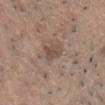The lesion was photographed on a routine skin check and not biopsied; there is no pathology result. About 2.5 mm across. Automated tile analysis of the lesion measured an average lesion color of about L≈48 a*≈15 b*≈24 (CIELAB) and a normalized lesion–skin contrast near 6. A male patient, aged approximately 45. Captured under white-light illumination. On the chest. A 15 mm crop from a total-body photograph taken for skin-cancer surveillance.